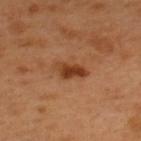Assessment: The lesion was photographed on a routine skin check and not biopsied; there is no pathology result. Acquisition and patient details: A lesion tile, about 15 mm wide, cut from a 3D total-body photograph. The patient is a male aged approximately 50. Located on the upper back. The tile uses cross-polarized illumination.